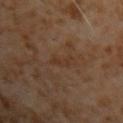Clinical summary: The lesion is located on the left upper arm. Captured under cross-polarized illumination. Automated image analysis of the tile measured a footprint of about 3 mm², an outline eccentricity of about 0.9 (0 = round, 1 = elongated), and two-axis asymmetry of about 0.55. Measured at roughly 3 mm in maximum diameter. A male subject, approximately 60 years of age. A lesion tile, about 15 mm wide, cut from a 3D total-body photograph.